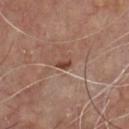follow-up=no biopsy performed (imaged during a skin exam)
acquisition=~15 mm crop, total-body skin-cancer survey
subject=male, aged 63 to 67
anatomic site=the chest